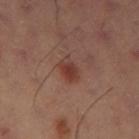{"biopsy_status": "not biopsied; imaged during a skin examination", "lesion_size": {"long_diameter_mm_approx": 3.0}, "image": {"source": "total-body photography crop", "field_of_view_mm": 15}, "site": "leg"}